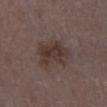Notes:
* follow-up: catalogued during a skin exam; not biopsied
* image: total-body-photography crop, ~15 mm field of view
* site: the leg
* automated metrics: an area of roughly 12 mm² and two-axis asymmetry of about 0.3; border irregularity of about 4 on a 0–10 scale, a color-variation rating of about 3.5/10, and a peripheral color-asymmetry measure near 1
* lighting: white-light
* patient: female, aged around 30
* diameter: about 5 mm